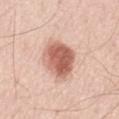This lesion was catalogued during total-body skin photography and was not selected for biopsy.
A roughly 15 mm field-of-view crop from a total-body skin photograph.
The total-body-photography lesion software estimated a footprint of about 15 mm² and an outline eccentricity of about 0.55 (0 = round, 1 = elongated). It also reported a lesion color around L≈61 a*≈25 b*≈29 in CIELAB, roughly 16 lightness units darker than nearby skin, and a normalized lesion–skin contrast near 10. It also reported border irregularity of about 1.5 on a 0–10 scale and internal color variation of about 5.5 on a 0–10 scale.
The lesion is on the lower back.
A male patient aged 68–72.
The recorded lesion diameter is about 4.5 mm.